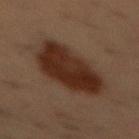Captured during whole-body skin photography for melanoma surveillance; the lesion was not biopsied. Captured under cross-polarized illumination. The lesion-visualizer software estimated a footprint of about 30 mm², an eccentricity of roughly 0.8, and a shape-asymmetry score of about 0.2 (0 = symmetric). The analysis additionally found an average lesion color of about L≈24 a*≈16 b*≈22 (CIELAB) and about 10 CIELAB-L* units darker than the surrounding skin. The software also gave a classifier nevus-likeness of about 95/100 and a lesion-detection confidence of about 100/100. Cropped from a total-body skin-imaging series; the visible field is about 15 mm. A male patient, in their mid- to late 50s. The lesion's longest dimension is about 8 mm. On the chest.